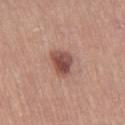Q: Was a biopsy performed?
A: total-body-photography surveillance lesion; no biopsy
Q: How was this image acquired?
A: ~15 mm crop, total-body skin-cancer survey
Q: How large is the lesion?
A: ~3.5 mm (longest diameter)
Q: What is the anatomic site?
A: the right thigh
Q: Illumination type?
A: white-light
Q: Patient demographics?
A: female, aged 63 to 67
Q: Automated lesion metrics?
A: border irregularity of about 2.5 on a 0–10 scale, a color-variation rating of about 5.5/10, and peripheral color asymmetry of about 2; an automated nevus-likeness rating near 90 out of 100